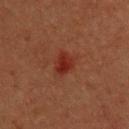No biopsy was performed on this lesion — it was imaged during a full skin examination and was not determined to be concerning. Imaged with cross-polarized lighting. The patient is a male approximately 40 years of age. A 15 mm close-up extracted from a 3D total-body photography capture. An algorithmic analysis of the crop reported border irregularity of about 2.5 on a 0–10 scale, a color-variation rating of about 2/10, and a peripheral color-asymmetry measure near 0.5. It also reported a nevus-likeness score of about 0/100 and lesion-presence confidence of about 100/100. Approximately 2.5 mm at its widest. Located on the upper back.